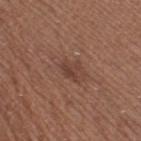follow-up: total-body-photography surveillance lesion; no biopsy
lighting: white-light
subject: female, aged around 65
anatomic site: the right thigh
automated lesion analysis: a footprint of about 3.5 mm², an outline eccentricity of about 0.5 (0 = round, 1 = elongated), and a shape-asymmetry score of about 0.45 (0 = symmetric); an average lesion color of about L≈40 a*≈21 b*≈25 (CIELAB), a lesion–skin lightness drop of about 7, and a normalized lesion–skin contrast near 6; a border-irregularity index near 4.5/10, internal color variation of about 1.5 on a 0–10 scale, and a peripheral color-asymmetry measure near 0.5; a classifier nevus-likeness of about 10/100 and lesion-presence confidence of about 100/100
imaging modality: total-body-photography crop, ~15 mm field of view